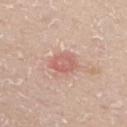workup: total-body-photography surveillance lesion; no biopsy
lighting: white-light
site: the chest
size: ≈3.5 mm
automated metrics: a mean CIELAB color near L≈61 a*≈25 b*≈25, a lesion–skin lightness drop of about 9, and a lesion-to-skin contrast of about 6 (normalized; higher = more distinct); a classifier nevus-likeness of about 50/100 and lesion-presence confidence of about 100/100
image source: total-body-photography crop, ~15 mm field of view
subject: male, approximately 60 years of age